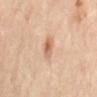The lesion was photographed on a routine skin check and not biopsied; there is no pathology result. From the mid back. A female subject aged 58 to 62. Captured under cross-polarized illumination. This image is a 15 mm lesion crop taken from a total-body photograph. Measured at roughly 2.5 mm in maximum diameter.